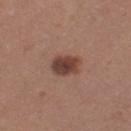Context: Cropped from a whole-body photographic skin survey; the tile spans about 15 mm. Automated image analysis of the tile measured a lesion area of about 7.5 mm², an outline eccentricity of about 0.75 (0 = round, 1 = elongated), and a shape-asymmetry score of about 0.2 (0 = symmetric). The recorded lesion diameter is about 4 mm. A female subject, in their mid- to late 30s. The lesion is located on the left thigh.